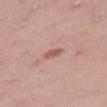Q: Was this lesion biopsied?
A: imaged on a skin check; not biopsied